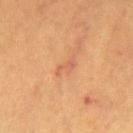The lesion was tiled from a total-body skin photograph and was not biopsied.
The patient is a female in their mid-40s.
Cropped from a whole-body photographic skin survey; the tile spans about 15 mm.
Measured at roughly 2.5 mm in maximum diameter.
The lesion is located on the right leg.
This is a cross-polarized tile.